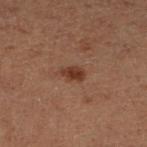Imaged during a routine full-body skin examination; the lesion was not biopsied and no histopathology is available.
The lesion's longest dimension is about 3 mm.
The lesion is on the right lower leg.
Automated tile analysis of the lesion measured a border-irregularity rating of about 2.5/10, a within-lesion color-variation index near 2/10, and peripheral color asymmetry of about 0.5. And it measured a nevus-likeness score of about 90/100 and lesion-presence confidence of about 100/100.
A male subject aged around 60.
A region of skin cropped from a whole-body photographic capture, roughly 15 mm wide.
Captured under cross-polarized illumination.